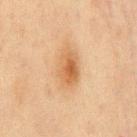{"lighting": "cross-polarized", "lesion_size": {"long_diameter_mm_approx": 4.5}, "automated_metrics": {"nevus_likeness_0_100": 95, "lesion_detection_confidence_0_100": 100}, "site": "chest", "patient": {"sex": "male", "age_approx": 45}, "image": {"source": "total-body photography crop", "field_of_view_mm": 15}}